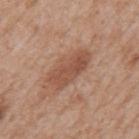Clinical impression:
The lesion was photographed on a routine skin check and not biopsied; there is no pathology result.
Background:
The recorded lesion diameter is about 5.5 mm. Cropped from a total-body skin-imaging series; the visible field is about 15 mm. Located on the back. Captured under white-light illumination. A male subject, in their mid- to late 60s.